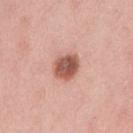This lesion was catalogued during total-body skin photography and was not selected for biopsy. The lesion is on the right upper arm. A female subject, in their mid-20s. Cropped from a whole-body photographic skin survey; the tile spans about 15 mm.